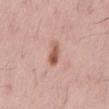biopsy status — no biopsy performed (imaged during a skin exam) | size — ~3 mm (longest diameter) | imaging modality — total-body-photography crop, ~15 mm field of view | tile lighting — white-light | site — the mid back | image-analysis metrics — a mean CIELAB color near L≈57 a*≈23 b*≈29, about 12 CIELAB-L* units darker than the surrounding skin, and a normalized border contrast of about 8.5; border irregularity of about 2 on a 0–10 scale, internal color variation of about 5.5 on a 0–10 scale, and radial color variation of about 2; a nevus-likeness score of about 90/100 | patient — male, about 65 years old.